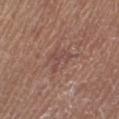Assessment:
Captured during whole-body skin photography for melanoma surveillance; the lesion was not biopsied.
Image and clinical context:
Longest diameter approximately 3 mm. A male subject, aged 63 to 67. Imaged with white-light lighting. Located on the right lower leg. A lesion tile, about 15 mm wide, cut from a 3D total-body photograph.